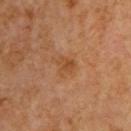– follow-up · catalogued during a skin exam; not biopsied
– automated lesion analysis · a lesion area of about 4.5 mm²; a lesion color around L≈47 a*≈23 b*≈36 in CIELAB, a lesion–skin lightness drop of about 6, and a normalized lesion–skin contrast near 6
– location · the chest
– size · ~2.5 mm (longest diameter)
– subject · male, about 60 years old
– illumination · cross-polarized illumination
– imaging modality · ~15 mm crop, total-body skin-cancer survey